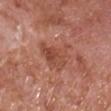Clinical impression: The lesion was tiled from a total-body skin photograph and was not biopsied. Image and clinical context: A lesion tile, about 15 mm wide, cut from a 3D total-body photograph. A male subject aged 63–67. The lesion is located on the front of the torso.